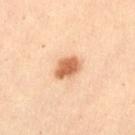follow-up = total-body-photography surveillance lesion; no biopsy
image-analysis metrics = a lesion color around L≈51 a*≈21 b*≈32 in CIELAB, about 13 CIELAB-L* units darker than the surrounding skin, and a normalized lesion–skin contrast near 9.5; a color-variation rating of about 2.5/10 and peripheral color asymmetry of about 1; a detector confidence of about 100 out of 100 that the crop contains a lesion
acquisition = ~15 mm crop, total-body skin-cancer survey
body site = the abdomen
diameter = ~3 mm (longest diameter)
subject = female, approximately 30 years of age
lighting = cross-polarized illumination